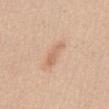| feature | finding |
|---|---|
| image-analysis metrics | a footprint of about 3.5 mm², an outline eccentricity of about 0.95 (0 = round, 1 = elongated), and a symmetry-axis asymmetry near 0.5; a lesion color around L≈64 a*≈20 b*≈33 in CIELAB, about 8 CIELAB-L* units darker than the surrounding skin, and a lesion-to-skin contrast of about 5.5 (normalized; higher = more distinct); a border-irregularity rating of about 5.5/10, a within-lesion color-variation index near 0.5/10, and a peripheral color-asymmetry measure near 0 |
| lesion size | ~3 mm (longest diameter) |
| lighting | white-light |
| patient | female, aged 38–42 |
| image source | ~15 mm crop, total-body skin-cancer survey |
| body site | the front of the torso |